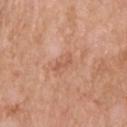{
  "biopsy_status": "not biopsied; imaged during a skin examination",
  "patient": {
    "sex": "male",
    "age_approx": 60
  },
  "image": {
    "source": "total-body photography crop",
    "field_of_view_mm": 15
  },
  "site": "upper back",
  "automated_metrics": {
    "cielab_L": 57,
    "cielab_a": 24,
    "cielab_b": 33,
    "vs_skin_darker_L": 7.0,
    "vs_skin_contrast_norm": 5.0
  }
}